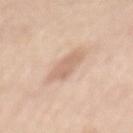Case summary:
- follow-up · no biopsy performed (imaged during a skin exam)
- body site · the mid back
- image source · total-body-photography crop, ~15 mm field of view
- lighting · white-light
- subject · female, aged 63–67
- TBP lesion metrics · about 9 CIELAB-L* units darker than the surrounding skin and a normalized lesion–skin contrast near 6; a border-irregularity index near 3/10, a color-variation rating of about 2/10, and radial color variation of about 0.5; a nevus-likeness score of about 5/100 and lesion-presence confidence of about 100/100
- lesion diameter · ≈4 mm